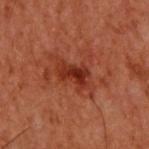workup = catalogued during a skin exam; not biopsied
diameter = about 4.5 mm
location = the upper back
acquisition = 15 mm crop, total-body photography
TBP lesion metrics = a classifier nevus-likeness of about 5/100 and a detector confidence of about 100 out of 100 that the crop contains a lesion
patient = male, aged around 60